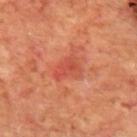{"biopsy_status": "not biopsied; imaged during a skin examination", "lesion_size": {"long_diameter_mm_approx": 3.0}, "patient": {"sex": "male", "age_approx": 70}, "image": {"source": "total-body photography crop", "field_of_view_mm": 15}, "lighting": "cross-polarized", "site": "mid back"}